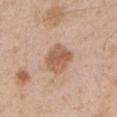| feature | finding |
|---|---|
| workup | catalogued during a skin exam; not biopsied |
| patient | male, approximately 50 years of age |
| imaging modality | ~15 mm tile from a whole-body skin photo |
| location | the arm |